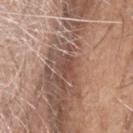biopsy status — imaged on a skin check; not biopsied
tile lighting — white-light
body site — the head or neck
diameter — ~2.5 mm (longest diameter)
image — total-body-photography crop, ~15 mm field of view
subject — male, aged around 75